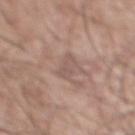Image and clinical context:
From the abdomen. An algorithmic analysis of the crop reported an eccentricity of roughly 0.9 and two-axis asymmetry of about 0.3. The software also gave a border-irregularity index near 3.5/10, internal color variation of about 1.5 on a 0–10 scale, and radial color variation of about 0.5. It also reported a classifier nevus-likeness of about 0/100 and a lesion-detection confidence of about 85/100. A male subject, about 70 years old. Imaged with white-light lighting. A roughly 15 mm field-of-view crop from a total-body skin photograph.